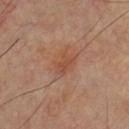Clinical impression: Captured during whole-body skin photography for melanoma surveillance; the lesion was not biopsied. Image and clinical context: The lesion-visualizer software estimated a lesion color around L≈48 a*≈23 b*≈31 in CIELAB, roughly 6 lightness units darker than nearby skin, and a normalized lesion–skin contrast near 5.5. It also reported a border-irregularity index near 3/10 and radial color variation of about 0.5. The analysis additionally found a nevus-likeness score of about 0/100 and lesion-presence confidence of about 100/100. A male subject aged approximately 50. A close-up tile cropped from a whole-body skin photograph, about 15 mm across. From the chest. Captured under cross-polarized illumination. The lesion's longest dimension is about 2.5 mm.